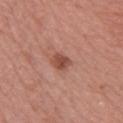follow-up: catalogued during a skin exam; not biopsied | illumination: white-light illumination | site: the front of the torso | lesion diameter: ~3 mm (longest diameter) | imaging modality: ~15 mm tile from a whole-body skin photo | patient: female, aged 68–72.